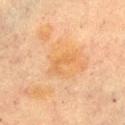{
  "biopsy_status": "not biopsied; imaged during a skin examination",
  "automated_metrics": {
    "vs_skin_darker_L": 5.0,
    "vs_skin_contrast_norm": 5.0,
    "border_irregularity_0_10": 5.0,
    "color_variation_0_10": 0.0,
    "nevus_likeness_0_100": 0
  },
  "lesion_size": {
    "long_diameter_mm_approx": 3.5
  },
  "patient": {
    "sex": "female",
    "age_approx": 60
  },
  "image": {
    "source": "total-body photography crop",
    "field_of_view_mm": 15
  },
  "lighting": "cross-polarized",
  "site": "chest"
}